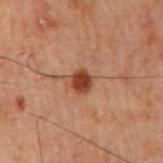Assessment: This lesion was catalogued during total-body skin photography and was not selected for biopsy. Image and clinical context: Imaged with cross-polarized lighting. Automated tile analysis of the lesion measured a shape eccentricity near 0.55 and two-axis asymmetry of about 0.15. It also reported a border-irregularity index near 1.5/10, a within-lesion color-variation index near 2/10, and peripheral color asymmetry of about 0.5. It also reported a classifier nevus-likeness of about 95/100 and a detector confidence of about 100 out of 100 that the crop contains a lesion. A male subject aged 58 to 62. The recorded lesion diameter is about 2.5 mm. On the right upper arm. A roughly 15 mm field-of-view crop from a total-body skin photograph.